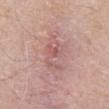No biopsy was performed on this lesion — it was imaged during a full skin examination and was not determined to be concerning. Approximately 5 mm at its widest. Located on the abdomen. This is a white-light tile. A 15 mm close-up tile from a total-body photography series done for melanoma screening. A male subject aged approximately 80.